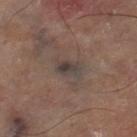Clinical impression: Captured during whole-body skin photography for melanoma surveillance; the lesion was not biopsied. Acquisition and patient details: This is a cross-polarized tile. Cropped from a total-body skin-imaging series; the visible field is about 15 mm. The lesion is located on the leg. Automated image analysis of the tile measured an area of roughly 4 mm², an eccentricity of roughly 0.75, and two-axis asymmetry of about 0.25. It also reported a mean CIELAB color near L≈39 a*≈11 b*≈16 and a normalized lesion–skin contrast near 8. And it measured a nevus-likeness score of about 0/100 and a lesion-detection confidence of about 60/100.